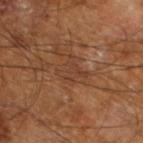| key | value |
|---|---|
| biopsy status | no biopsy performed (imaged during a skin exam) |
| acquisition | ~15 mm tile from a whole-body skin photo |
| body site | the left lower leg |
| subject | male, aged 63 to 67 |